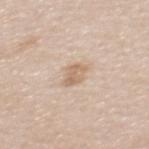Captured during whole-body skin photography for melanoma surveillance; the lesion was not biopsied.
The tile uses white-light illumination.
A female subject aged around 45.
Located on the mid back.
The lesion-visualizer software estimated a classifier nevus-likeness of about 15/100 and lesion-presence confidence of about 100/100.
A roughly 15 mm field-of-view crop from a total-body skin photograph.